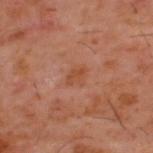biopsy_status: not biopsied; imaged during a skin examination
site: upper back
lighting: cross-polarized
image:
  source: total-body photography crop
  field_of_view_mm: 15
lesion_size:
  long_diameter_mm_approx: 2.5
automated_metrics:
  area_mm2_approx: 4.5
  eccentricity: 0.7
  nevus_likeness_0_100: 0
  lesion_detection_confidence_0_100: 100
patient:
  sex: male
  age_approx: 60